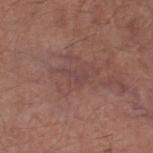Impression: Imaged during a routine full-body skin examination; the lesion was not biopsied and no histopathology is available. Clinical summary: Measured at roughly 2.5 mm in maximum diameter. Automated tile analysis of the lesion measured an area of roughly 2.5 mm². It also reported an average lesion color of about L≈43 a*≈20 b*≈20 (CIELAB), roughly 5 lightness units darker than nearby skin, and a normalized border contrast of about 4.5. It also reported an automated nevus-likeness rating near 0 out of 100 and a lesion-detection confidence of about 80/100. The subject is a male aged 63–67. The lesion is located on the right thigh. A 15 mm close-up extracted from a 3D total-body photography capture. Imaged with white-light lighting.